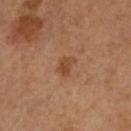This lesion was catalogued during total-body skin photography and was not selected for biopsy. Approximately 2.5 mm at its widest. A close-up tile cropped from a whole-body skin photograph, about 15 mm across. Located on the left lower leg. A female patient, in their mid-60s. An algorithmic analysis of the crop reported an automated nevus-likeness rating near 15 out of 100 and a detector confidence of about 100 out of 100 that the crop contains a lesion.